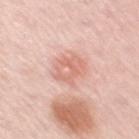The lesion was photographed on a routine skin check and not biopsied; there is no pathology result. An algorithmic analysis of the crop reported a shape eccentricity near 0.45 and a shape-asymmetry score of about 0.25 (0 = symmetric). And it measured an average lesion color of about L≈68 a*≈24 b*≈28 (CIELAB), a lesion–skin lightness drop of about 9, and a normalized lesion–skin contrast near 5.5. The software also gave a border-irregularity index near 3/10 and a peripheral color-asymmetry measure near 2. The subject is a female aged approximately 50. On the right upper arm. Imaged with white-light lighting. Approximately 4 mm at its widest. A lesion tile, about 15 mm wide, cut from a 3D total-body photograph.